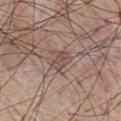Assessment:
The lesion was photographed on a routine skin check and not biopsied; there is no pathology result.
Background:
From the chest. A male patient in their mid- to late 70s. Approximately 3 mm at its widest. The lesion-visualizer software estimated a footprint of about 4.5 mm² and an outline eccentricity of about 0.75 (0 = round, 1 = elongated). And it measured a border-irregularity index near 2.5/10, a within-lesion color-variation index near 2/10, and a peripheral color-asymmetry measure near 1. It also reported an automated nevus-likeness rating near 0 out of 100 and a lesion-detection confidence of about 100/100. The tile uses white-light illumination. A close-up tile cropped from a whole-body skin photograph, about 15 mm across.